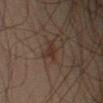| key | value |
|---|---|
| lesion diameter | ≈2.5 mm |
| tile lighting | cross-polarized illumination |
| patient | male, roughly 50 years of age |
| image | 15 mm crop, total-body photography |
| automated metrics | an outline eccentricity of about 0.85 (0 = round, 1 = elongated) and a symmetry-axis asymmetry near 0.2; a lesion color around L≈22 a*≈13 b*≈18 in CIELAB, a lesion–skin lightness drop of about 5, and a lesion-to-skin contrast of about 6.5 (normalized; higher = more distinct); a detector confidence of about 100 out of 100 that the crop contains a lesion |
| site | the left forearm |